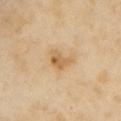About 3 mm across.
Imaged with cross-polarized lighting.
A female patient about 55 years old.
A close-up tile cropped from a whole-body skin photograph, about 15 mm across.
On the chest.